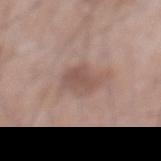Part of a total-body skin-imaging series; this lesion was reviewed on a skin check and was not flagged for biopsy.